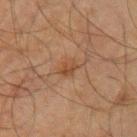No biopsy was performed on this lesion — it was imaged during a full skin examination and was not determined to be concerning.
About 2.5 mm across.
A region of skin cropped from a whole-body photographic capture, roughly 15 mm wide.
The lesion-visualizer software estimated an outline eccentricity of about 0.8 (0 = round, 1 = elongated) and a shape-asymmetry score of about 0.3 (0 = symmetric). And it measured border irregularity of about 2.5 on a 0–10 scale, internal color variation of about 3 on a 0–10 scale, and a peripheral color-asymmetry measure near 1.5.
A male patient aged approximately 65.
From the arm.